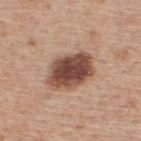Recorded during total-body skin imaging; not selected for excision or biopsy.
Captured under white-light illumination.
The lesion's longest dimension is about 5.5 mm.
A 15 mm crop from a total-body photograph taken for skin-cancer surveillance.
The lesion is located on the upper back.
The subject is a male aged 58 to 62.